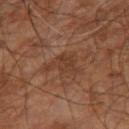The patient is a male aged 58 to 62. A 15 mm close-up tile from a total-body photography series done for melanoma screening. Located on the right leg.